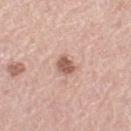The lesion was photographed on a routine skin check and not biopsied; there is no pathology result. Captured under white-light illumination. A 15 mm crop from a total-body photograph taken for skin-cancer surveillance. The subject is a female aged 63 to 67. On the left thigh.